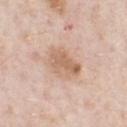  biopsy_status: not biopsied; imaged during a skin examination
  automated_metrics:
    border_irregularity_0_10: 2.5
    color_variation_0_10: 4.5
    peripheral_color_asymmetry: 1.5
  lighting: white-light
  image:
    source: total-body photography crop
    field_of_view_mm: 15
  lesion_size:
    long_diameter_mm_approx: 4.5
  site: chest
  patient:
    sex: male
    age_approx: 60